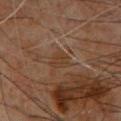follow-up: no biopsy performed (imaged during a skin exam) | tile lighting: cross-polarized illumination | imaging modality: ~15 mm tile from a whole-body skin photo | automated lesion analysis: an automated nevus-likeness rating near 0 out of 100 and a lesion-detection confidence of about 75/100 | lesion size: ~2.5 mm (longest diameter) | location: the upper back | patient: male, roughly 60 years of age.